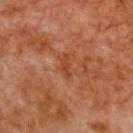<case>
<biopsy_status>not biopsied; imaged during a skin examination</biopsy_status>
<image>
  <source>total-body photography crop</source>
  <field_of_view_mm>15</field_of_view_mm>
</image>
<site>chest</site>
<patient>
  <sex>male</sex>
  <age_approx>80</age_approx>
</patient>
<lesion_size>
  <long_diameter_mm_approx>2.5</long_diameter_mm_approx>
</lesion_size>
<lighting>cross-polarized</lighting>
</case>